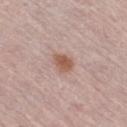biopsy status: no biopsy performed (imaged during a skin exam) | lighting: white-light | acquisition: 15 mm crop, total-body photography | diameter: ~2.5 mm (longest diameter) | body site: the left leg | patient: female, aged approximately 65 | image-analysis metrics: a lesion area of about 4.5 mm², an outline eccentricity of about 0.55 (0 = round, 1 = elongated), and a shape-asymmetry score of about 0.2 (0 = symmetric); about 11 CIELAB-L* units darker than the surrounding skin and a normalized lesion–skin contrast near 8.5.